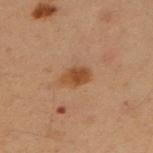Q: Was this lesion biopsied?
A: total-body-photography surveillance lesion; no biopsy
Q: What are the patient's age and sex?
A: female, aged approximately 50
Q: What is the imaging modality?
A: 15 mm crop, total-body photography
Q: Lesion size?
A: about 3.5 mm
Q: What lighting was used for the tile?
A: cross-polarized
Q: Lesion location?
A: the arm